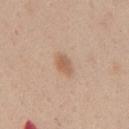• biopsy status: no biopsy performed (imaged during a skin exam)
• image source: ~15 mm tile from a whole-body skin photo
• image-analysis metrics: a lesion color around L≈59 a*≈19 b*≈31 in CIELAB and a normalized border contrast of about 7
• lesion size: ≈2.5 mm
• lighting: white-light
• patient: female, about 40 years old
• location: the back